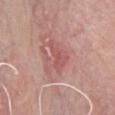| field | value |
|---|---|
| notes | imaged on a skin check; not biopsied |
| lesion diameter | about 3.5 mm |
| image source | 15 mm crop, total-body photography |
| subject | male, roughly 65 years of age |
| location | the chest |
| illumination | white-light illumination |